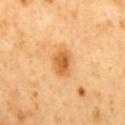workup — imaged on a skin check; not biopsied
imaging modality — ~15 mm tile from a whole-body skin photo
illumination — cross-polarized
body site — the mid back
patient — male, about 50 years old
automated lesion analysis — a lesion area of about 6.5 mm², an outline eccentricity of about 0.7 (0 = round, 1 = elongated), and a symmetry-axis asymmetry near 0.2; a border-irregularity rating of about 2/10, a within-lesion color-variation index near 4/10, and radial color variation of about 1.5; a nevus-likeness score of about 85/100 and a lesion-detection confidence of about 100/100